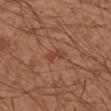Impression:
Imaged during a routine full-body skin examination; the lesion was not biopsied and no histopathology is available.
Background:
This is a white-light tile. A male patient in their 30s. Located on the right forearm. Automated image analysis of the tile measured a footprint of about 3 mm² and an eccentricity of roughly 0.8. It also reported a lesion color around L≈43 a*≈24 b*≈29 in CIELAB, about 7 CIELAB-L* units darker than the surrounding skin, and a normalized border contrast of about 5.5. The software also gave a lesion-detection confidence of about 100/100. This image is a 15 mm lesion crop taken from a total-body photograph.